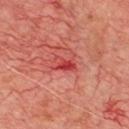| feature | finding |
|---|---|
| follow-up | total-body-photography surveillance lesion; no biopsy |
| TBP lesion metrics | a lesion color around L≈46 a*≈41 b*≈29 in CIELAB, about 10 CIELAB-L* units darker than the surrounding skin, and a lesion-to-skin contrast of about 7 (normalized; higher = more distinct); a border-irregularity rating of about 4/10 and peripheral color asymmetry of about 1; lesion-presence confidence of about 85/100 |
| lesion diameter | ~3 mm (longest diameter) |
| anatomic site | the chest |
| acquisition | ~15 mm crop, total-body skin-cancer survey |
| patient | male, roughly 65 years of age |